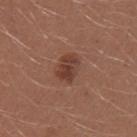Assessment: This lesion was catalogued during total-body skin photography and was not selected for biopsy. Image and clinical context: The lesion-visualizer software estimated a lesion color around L≈40 a*≈22 b*≈26 in CIELAB and about 9 CIELAB-L* units darker than the surrounding skin. Imaged with white-light lighting. Cropped from a total-body skin-imaging series; the visible field is about 15 mm. The subject is a female aged approximately 25. The lesion is on the left upper arm. Longest diameter approximately 3.5 mm.